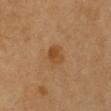Case summary:
- biopsy status: no biopsy performed (imaged during a skin exam)
- patient: female, aged 63 to 67
- image source: ~15 mm crop, total-body skin-cancer survey
- diameter: ~2.5 mm (longest diameter)
- illumination: cross-polarized illumination
- site: the chest